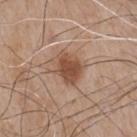notes: no biopsy performed (imaged during a skin exam)